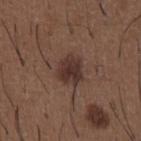biopsy_status: not biopsied; imaged during a skin examination
patient:
  sex: male
  age_approx: 50
lesion_size:
  long_diameter_mm_approx: 3.5
lighting: white-light
image:
  source: total-body photography crop
  field_of_view_mm: 15
automated_metrics:
  nevus_likeness_0_100: 85
  lesion_detection_confidence_0_100: 100
site: chest